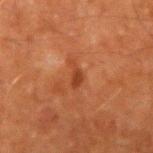| field | value |
|---|---|
| workup | total-body-photography surveillance lesion; no biopsy |
| acquisition | total-body-photography crop, ~15 mm field of view |
| body site | the right upper arm |
| subject | male, about 60 years old |